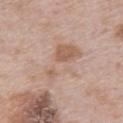Impression:
The lesion was photographed on a routine skin check and not biopsied; there is no pathology result.
Context:
An algorithmic analysis of the crop reported an outline eccentricity of about 0.85 (0 = round, 1 = elongated) and a shape-asymmetry score of about 0.45 (0 = symmetric). The software also gave an average lesion color of about L≈61 a*≈17 b*≈27 (CIELAB), roughly 7 lightness units darker than nearby skin, and a normalized border contrast of about 5. The software also gave border irregularity of about 6.5 on a 0–10 scale and radial color variation of about 2. This is a white-light tile. A 15 mm crop from a total-body photograph taken for skin-cancer surveillance. The lesion's longest dimension is about 6.5 mm. On the back. A female patient aged 63 to 67.